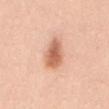| field | value |
|---|---|
| biopsy status | imaged on a skin check; not biopsied |
| diameter | ≈4.5 mm |
| automated lesion analysis | an area of roughly 8.5 mm², an outline eccentricity of about 0.85 (0 = round, 1 = elongated), and a symmetry-axis asymmetry near 0.25; a lesion–skin lightness drop of about 14 and a normalized lesion–skin contrast near 8.5; a border-irregularity index near 2.5/10 and internal color variation of about 3.5 on a 0–10 scale |
| lighting | white-light |
| imaging modality | ~15 mm tile from a whole-body skin photo |
| site | the mid back |
| patient | female, aged approximately 40 |